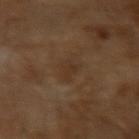The patient is a male aged 63 to 67.
Cropped from a total-body skin-imaging series; the visible field is about 15 mm.
Longest diameter approximately 3 mm.